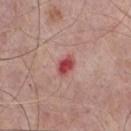biopsy status — imaged on a skin check; not biopsied | imaging modality — ~15 mm crop, total-body skin-cancer survey | body site — the chest | lesion size — about 2.5 mm | subject — male, aged approximately 75 | illumination — white-light illumination.